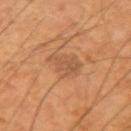<case>
<biopsy_status>not biopsied; imaged during a skin examination</biopsy_status>
<image>
  <source>total-body photography crop</source>
  <field_of_view_mm>15</field_of_view_mm>
</image>
<lighting>cross-polarized</lighting>
<site>right upper arm</site>
<lesion_size>
  <long_diameter_mm_approx>3.5</long_diameter_mm_approx>
</lesion_size>
<patient>
  <sex>male</sex>
  <age_approx>55</age_approx>
</patient>
<automated_metrics>
  <cielab_L>49</cielab_L>
  <cielab_a>20</cielab_a>
  <cielab_b>33</cielab_b>
  <vs_skin_darker_L>6.0</vs_skin_darker_L>
  <border_irregularity_0_10>4.5</border_irregularity_0_10>
  <color_variation_0_10>2.0</color_variation_0_10>
  <peripheral_color_asymmetry>1.0</peripheral_color_asymmetry>
  <lesion_detection_confidence_0_100>100</lesion_detection_confidence_0_100>
</automated_metrics>
</case>